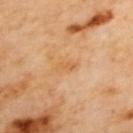No biopsy was performed on this lesion — it was imaged during a full skin examination and was not determined to be concerning. Captured under cross-polarized illumination. An algorithmic analysis of the crop reported a footprint of about 3 mm², an eccentricity of roughly 0.9, and two-axis asymmetry of about 0.3. And it measured a lesion color around L≈64 a*≈23 b*≈44 in CIELAB, a lesion–skin lightness drop of about 6, and a normalized lesion–skin contrast near 5. The analysis additionally found internal color variation of about 0 on a 0–10 scale and peripheral color asymmetry of about 0. Cropped from a whole-body photographic skin survey; the tile spans about 15 mm. About 2.5 mm across. From the upper back.